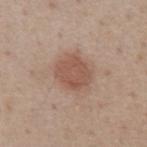<record>
<biopsy_status>not biopsied; imaged during a skin examination</biopsy_status>
<image>
  <source>total-body photography crop</source>
  <field_of_view_mm>15</field_of_view_mm>
</image>
<patient>
  <sex>male</sex>
  <age_approx>60</age_approx>
</patient>
<site>front of the torso</site>
<lesion_size>
  <long_diameter_mm_approx>4.0</long_diameter_mm_approx>
</lesion_size>
<automated_metrics>
  <area_mm2_approx>11.0</area_mm2_approx>
  <eccentricity>0.45</eccentricity>
  <nevus_likeness_0_100>90</nevus_likeness_0_100>
  <lesion_detection_confidence_0_100>100</lesion_detection_confidence_0_100>
</automated_metrics>
<lighting>white-light</lighting>
</record>